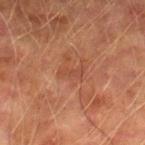{
  "biopsy_status": "not biopsied; imaged during a skin examination",
  "patient": {
    "sex": "male",
    "age_approx": 75
  },
  "lighting": "cross-polarized",
  "image": {
    "source": "total-body photography crop",
    "field_of_view_mm": 15
  },
  "lesion_size": {
    "long_diameter_mm_approx": 3.0
  },
  "site": "right lower leg"
}